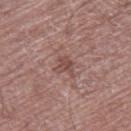No biopsy was performed on this lesion — it was imaged during a full skin examination and was not determined to be concerning.
Automated tile analysis of the lesion measured an area of roughly 4 mm², an eccentricity of roughly 0.65, and two-axis asymmetry of about 0.35. The software also gave roughly 8 lightness units darker than nearby skin and a lesion-to-skin contrast of about 6.5 (normalized; higher = more distinct).
The recorded lesion diameter is about 2.5 mm.
Imaged with white-light lighting.
A male subject, aged around 75.
Located on the right thigh.
This image is a 15 mm lesion crop taken from a total-body photograph.